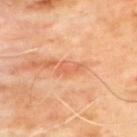Q: Was a biopsy performed?
A: imaged on a skin check; not biopsied
Q: Patient demographics?
A: male, aged around 65
Q: What is the imaging modality?
A: 15 mm crop, total-body photography
Q: What is the anatomic site?
A: the back